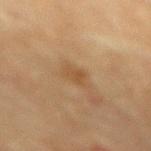Acquisition and patient details: The subject is a female aged approximately 80. The tile uses cross-polarized illumination. On the mid back. The lesion-visualizer software estimated a lesion area of about 3.5 mm², a shape eccentricity near 0.75, and two-axis asymmetry of about 0.3. Cropped from a total-body skin-imaging series; the visible field is about 15 mm.